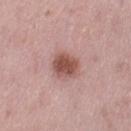Q: Was a biopsy performed?
A: total-body-photography surveillance lesion; no biopsy
Q: What did automated image analysis measure?
A: a lesion area of about 7.5 mm², an outline eccentricity of about 0.5 (0 = round, 1 = elongated), and two-axis asymmetry of about 0.15; a lesion color around L≈51 a*≈24 b*≈24 in CIELAB and about 14 CIELAB-L* units darker than the surrounding skin; a border-irregularity index near 1.5/10, internal color variation of about 3.5 on a 0–10 scale, and peripheral color asymmetry of about 1
Q: Lesion location?
A: the left thigh
Q: What are the patient's age and sex?
A: female, in their 50s
Q: How was the tile lit?
A: white-light
Q: How was this image acquired?
A: ~15 mm crop, total-body skin-cancer survey